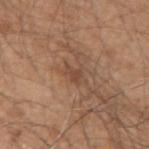Captured during whole-body skin photography for melanoma surveillance; the lesion was not biopsied. About 3.5 mm across. Captured under white-light illumination. From the right upper arm. Cropped from a total-body skin-imaging series; the visible field is about 15 mm. An algorithmic analysis of the crop reported an area of roughly 4.5 mm², a shape eccentricity near 0.8, and a shape-asymmetry score of about 0.4 (0 = symmetric). The analysis additionally found an average lesion color of about L≈47 a*≈19 b*≈29 (CIELAB) and a lesion–skin lightness drop of about 7. A male subject aged 48–52.